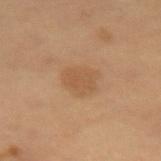Clinical impression: No biopsy was performed on this lesion — it was imaged during a full skin examination and was not determined to be concerning. Image and clinical context: The tile uses cross-polarized illumination. A male subject roughly 60 years of age. The recorded lesion diameter is about 3.5 mm. An algorithmic analysis of the crop reported a lesion–skin lightness drop of about 6 and a normalized border contrast of about 5. And it measured a border-irregularity index near 2/10, internal color variation of about 2 on a 0–10 scale, and a peripheral color-asymmetry measure near 0.5. The analysis additionally found a lesion-detection confidence of about 100/100. Located on the left thigh. Cropped from a whole-body photographic skin survey; the tile spans about 15 mm.